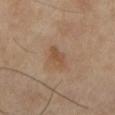Findings:
* workup: catalogued during a skin exam; not biopsied
* subject: female, about 70 years old
* lighting: cross-polarized
* anatomic site: the left lower leg
* imaging modality: ~15 mm tile from a whole-body skin photo
* automated metrics: a shape eccentricity near 0.75; an average lesion color of about L≈51 a*≈18 b*≈32 (CIELAB), a lesion–skin lightness drop of about 7, and a normalized border contrast of about 6.5